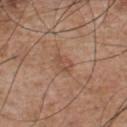Case summary:
• notes — total-body-photography surveillance lesion; no biopsy
• lighting — white-light
• subject — male, aged approximately 55
• image — 15 mm crop, total-body photography
• size — ~3 mm (longest diameter)
• location — the chest